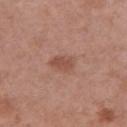The lesion was tiled from a total-body skin photograph and was not biopsied.
The lesion's longest dimension is about 3 mm.
The lesion is located on the chest.
This image is a 15 mm lesion crop taken from a total-body photograph.
The patient is a female about 40 years old.